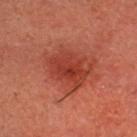This lesion was catalogued during total-body skin photography and was not selected for biopsy.
Measured at roughly 4.5 mm in maximum diameter.
Cropped from a whole-body photographic skin survey; the tile spans about 15 mm.
The lesion is on the head or neck.
The tile uses cross-polarized illumination.
A male subject, aged around 50.